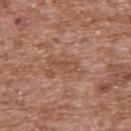The lesion was tiled from a total-body skin photograph and was not biopsied.
Automated image analysis of the tile measured an eccentricity of roughly 0.9 and two-axis asymmetry of about 0.45. The software also gave a border-irregularity rating of about 5/10, a within-lesion color-variation index near 1.5/10, and peripheral color asymmetry of about 0. The software also gave a nevus-likeness score of about 0/100 and a lesion-detection confidence of about 95/100.
Located on the upper back.
This is a white-light tile.
The patient is a male aged approximately 45.
A 15 mm crop from a total-body photograph taken for skin-cancer surveillance.
Approximately 3.5 mm at its widest.